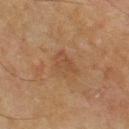{
  "biopsy_status": "not biopsied; imaged during a skin examination",
  "site": "upper back",
  "lighting": "cross-polarized",
  "lesion_size": {
    "long_diameter_mm_approx": 3.0
  },
  "patient": {
    "sex": "male",
    "age_approx": 70
  },
  "automated_metrics": {
    "vs_skin_darker_L": 5.0,
    "vs_skin_contrast_norm": 4.5,
    "border_irregularity_0_10": 3.0,
    "peripheral_color_asymmetry": 0.5
  },
  "image": {
    "source": "total-body photography crop",
    "field_of_view_mm": 15
  }
}